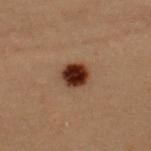workup = catalogued during a skin exam; not biopsied | subject = female, aged approximately 30 | acquisition = total-body-photography crop, ~15 mm field of view | site = the upper back | diameter = about 3 mm.